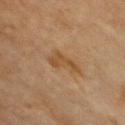notes: imaged on a skin check; not biopsied
size: about 3.5 mm
body site: the chest
lighting: cross-polarized
TBP lesion metrics: roughly 7 lightness units darker than nearby skin and a lesion-to-skin contrast of about 7 (normalized; higher = more distinct); border irregularity of about 5.5 on a 0–10 scale and peripheral color asymmetry of about 0.5
patient: female, in their 60s
image: ~15 mm crop, total-body skin-cancer survey